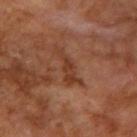Impression:
Recorded during total-body skin imaging; not selected for excision or biopsy.
Acquisition and patient details:
The patient is a male roughly 55 years of age. A 15 mm close-up extracted from a 3D total-body photography capture. Located on the right upper arm.